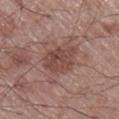Captured during whole-body skin photography for melanoma surveillance; the lesion was not biopsied. Imaged with white-light lighting. On the right lower leg. Cropped from a total-body skin-imaging series; the visible field is about 15 mm. A male patient, aged around 70.